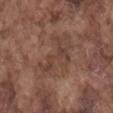notes — no biopsy performed (imaged during a skin exam)